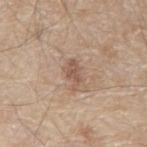{
  "biopsy_status": "not biopsied; imaged during a skin examination",
  "lighting": "white-light",
  "image": {
    "source": "total-body photography crop",
    "field_of_view_mm": 15
  },
  "lesion_size": {
    "long_diameter_mm_approx": 3.0
  },
  "site": "back",
  "patient": {
    "sex": "male",
    "age_approx": 80
  },
  "automated_metrics": {
    "cielab_L": 54,
    "cielab_a": 18,
    "cielab_b": 27,
    "vs_skin_contrast_norm": 7.0,
    "border_irregularity_0_10": 4.0,
    "color_variation_0_10": 1.5,
    "peripheral_color_asymmetry": 0.5,
    "nevus_likeness_0_100": 0,
    "lesion_detection_confidence_0_100": 100
  }
}